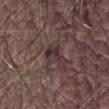Clinical impression:
Imaged during a routine full-body skin examination; the lesion was not biopsied and no histopathology is available.
Acquisition and patient details:
The total-body-photography lesion software estimated a lesion area of about 5.5 mm² and an outline eccentricity of about 0.65 (0 = round, 1 = elongated). A region of skin cropped from a whole-body photographic capture, roughly 15 mm wide. This is a white-light tile. The lesion is located on the leg. The recorded lesion diameter is about 3 mm. The patient is a male aged 48–52.